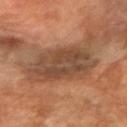Recorded during total-body skin imaging; not selected for excision or biopsy.
Located on the right forearm.
The tile uses cross-polarized illumination.
A region of skin cropped from a whole-body photographic capture, roughly 15 mm wide.
A female patient, roughly 70 years of age.